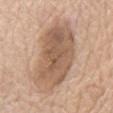Findings:
- follow-up — catalogued during a skin exam; not biopsied
- lesion diameter — ≈9.5 mm
- illumination — white-light illumination
- site — the front of the torso
- subject — male, aged approximately 75
- acquisition — ~15 mm crop, total-body skin-cancer survey